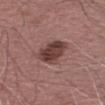Longest diameter approximately 4 mm. Automated image analysis of the tile measured a lesion–skin lightness drop of about 13. It also reported a border-irregularity rating of about 2.5/10, a within-lesion color-variation index near 4/10, and radial color variation of about 1.5. From the arm. A male subject, aged 68 to 72. This image is a 15 mm lesion crop taken from a total-body photograph.